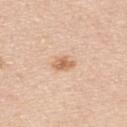biopsy status: imaged on a skin check; not biopsied | tile lighting: white-light illumination | subject: male, in their mid-40s | lesion diameter: about 3 mm | TBP lesion metrics: an eccentricity of roughly 0.8 and a shape-asymmetry score of about 0.2 (0 = symmetric); about 11 CIELAB-L* units darker than the surrounding skin and a normalized lesion–skin contrast near 7.5; border irregularity of about 2 on a 0–10 scale, internal color variation of about 2.5 on a 0–10 scale, and peripheral color asymmetry of about 0.5 | location: the upper back | imaging modality: 15 mm crop, total-body photography.